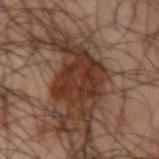The lesion was photographed on a routine skin check and not biopsied; there is no pathology result. A male patient in their 50s. Automated tile analysis of the lesion measured an area of roughly 23 mm², an outline eccentricity of about 0.85 (0 = round, 1 = elongated), and two-axis asymmetry of about 0.4. It also reported a border-irregularity rating of about 7/10 and a color-variation rating of about 3.5/10. The software also gave a nevus-likeness score of about 35/100 and a lesion-detection confidence of about 90/100. A 15 mm crop from a total-body photograph taken for skin-cancer surveillance. From the left upper arm. The recorded lesion diameter is about 9 mm.